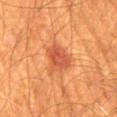biopsy status = catalogued during a skin exam; not biopsied
subject = male, aged 63 to 67
TBP lesion metrics = an average lesion color of about L≈51 a*≈30 b*≈38 (CIELAB), a lesion–skin lightness drop of about 10, and a normalized border contrast of about 7; border irregularity of about 3 on a 0–10 scale, internal color variation of about 3.5 on a 0–10 scale, and radial color variation of about 1
lesion diameter = about 4 mm
image source = total-body-photography crop, ~15 mm field of view
location = the mid back